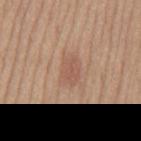<tbp_lesion>
  <biopsy_status>not biopsied; imaged during a skin examination</biopsy_status>
  <lesion_size>
    <long_diameter_mm_approx>3.5</long_diameter_mm_approx>
  </lesion_size>
  <image>
    <source>total-body photography crop</source>
    <field_of_view_mm>15</field_of_view_mm>
  </image>
  <lighting>white-light</lighting>
  <site>mid back</site>
  <automated_metrics>
    <cielab_L>55</cielab_L>
    <cielab_a>20</cielab_a>
    <cielab_b>28</cielab_b>
    <vs_skin_darker_L>7.0</vs_skin_darker_L>
    <border_irregularity_0_10>3.0</border_irregularity_0_10>
    <color_variation_0_10>2.0</color_variation_0_10>
    <peripheral_color_asymmetry>1.0</peripheral_color_asymmetry>
  </automated_metrics>
  <patient>
    <sex>male</sex>
    <age_approx>60</age_approx>
  </patient>
</tbp_lesion>